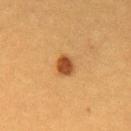The lesion was tiled from a total-body skin photograph and was not biopsied.
The subject is a female approximately 30 years of age.
Imaged with cross-polarized lighting.
On the front of the torso.
The total-body-photography lesion software estimated a lesion area of about 4 mm² and a shape-asymmetry score of about 0.2 (0 = symmetric). The analysis additionally found an average lesion color of about L≈46 a*≈25 b*≈40 (CIELAB) and a normalized lesion–skin contrast near 10.5. It also reported an automated nevus-likeness rating near 100 out of 100 and lesion-presence confidence of about 100/100.
Cropped from a whole-body photographic skin survey; the tile spans about 15 mm.